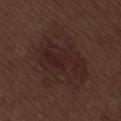| field | value |
|---|---|
| size | ≈10 mm |
| patient | male, approximately 70 years of age |
| image | 15 mm crop, total-body photography |
| lighting | white-light illumination |
| body site | the lower back |
| TBP lesion metrics | an area of roughly 39 mm², an outline eccentricity of about 0.75 (0 = round, 1 = elongated), and two-axis asymmetry of about 0.3; a lesion color around L≈24 a*≈16 b*≈19 in CIELAB and roughly 5 lightness units darker than nearby skin; border irregularity of about 4 on a 0–10 scale and internal color variation of about 3.5 on a 0–10 scale |